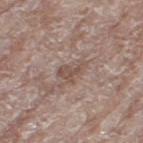Captured during whole-body skin photography for melanoma surveillance; the lesion was not biopsied. A 15 mm crop from a total-body photograph taken for skin-cancer surveillance. The tile uses white-light illumination. An algorithmic analysis of the crop reported an area of roughly 4.5 mm² and a symmetry-axis asymmetry near 0.45. It also reported a lesion color around L≈50 a*≈17 b*≈23 in CIELAB and roughly 8 lightness units darker than nearby skin. The analysis additionally found lesion-presence confidence of about 100/100. A female subject in their mid-70s. On the leg. About 3.5 mm across.